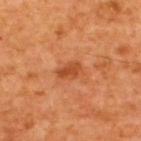Findings:
• biopsy status: imaged on a skin check; not biopsied
• diameter: about 3 mm
• illumination: cross-polarized
• patient: male, aged around 65
• imaging modality: 15 mm crop, total-body photography
• anatomic site: the upper back